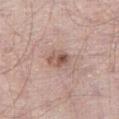Captured during whole-body skin photography for melanoma surveillance; the lesion was not biopsied. The patient is a male approximately 65 years of age. About 3 mm across. A 15 mm close-up extracted from a 3D total-body photography capture. The lesion is on the right thigh. Automated tile analysis of the lesion measured a within-lesion color-variation index near 8/10 and peripheral color asymmetry of about 3.5. This is a white-light tile.